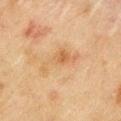workup: catalogued during a skin exam; not biopsied
subject: male, aged 43–47
lesion diameter: about 5.5 mm
body site: the left upper arm
tile lighting: cross-polarized illumination
imaging modality: 15 mm crop, total-body photography